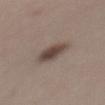Impression:
Captured during whole-body skin photography for melanoma surveillance; the lesion was not biopsied.
Clinical summary:
Approximately 4.5 mm at its widest. The lesion is located on the back. A close-up tile cropped from a whole-body skin photograph, about 15 mm across. The subject is a female in their mid- to late 40s.